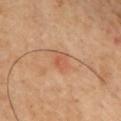Q: What kind of image is this?
A: 15 mm crop, total-body photography
Q: Patient demographics?
A: male, about 55 years old
Q: What is the anatomic site?
A: the front of the torso